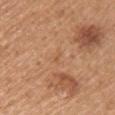Q: Is there a histopathology result?
A: catalogued during a skin exam; not biopsied
Q: Patient demographics?
A: female, about 55 years old
Q: How was this image acquired?
A: ~15 mm crop, total-body skin-cancer survey
Q: What is the lesion's diameter?
A: about 2 mm
Q: What is the anatomic site?
A: the right upper arm
Q: What lighting was used for the tile?
A: white-light